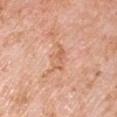Captured during whole-body skin photography for melanoma surveillance; the lesion was not biopsied.
On the right upper arm.
A male subject approximately 60 years of age.
Measured at roughly 3.5 mm in maximum diameter.
A region of skin cropped from a whole-body photographic capture, roughly 15 mm wide.